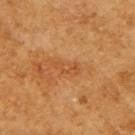<case>
<biopsy_status>not biopsied; imaged during a skin examination</biopsy_status>
<lesion_size>
  <long_diameter_mm_approx>3.0</long_diameter_mm_approx>
</lesion_size>
<image>
  <source>total-body photography crop</source>
  <field_of_view_mm>15</field_of_view_mm>
</image>
<site>right upper arm</site>
<patient>
  <sex>female</sex>
  <age_approx>55</age_approx>
</patient>
<lighting>cross-polarized</lighting>
</case>